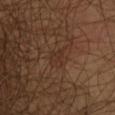<lesion>
<biopsy_status>not biopsied; imaged during a skin examination</biopsy_status>
<lesion_size>
  <long_diameter_mm_approx>2.5</long_diameter_mm_approx>
</lesion_size>
<image>
  <source>total-body photography crop</source>
  <field_of_view_mm>15</field_of_view_mm>
</image>
<patient>
  <sex>male</sex>
  <age_approx>45</age_approx>
</patient>
<lighting>cross-polarized</lighting>
<site>chest</site>
</lesion>